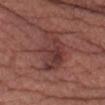Context:
About 5 mm across. The patient is a female approximately 60 years of age. Imaged with white-light lighting. A 15 mm crop from a total-body photograph taken for skin-cancer surveillance. An algorithmic analysis of the crop reported a footprint of about 13 mm² and a symmetry-axis asymmetry near 0.45. And it measured about 8 CIELAB-L* units darker than the surrounding skin and a normalized border contrast of about 7. The analysis additionally found internal color variation of about 4.5 on a 0–10 scale and peripheral color asymmetry of about 1.5. The analysis additionally found a classifier nevus-likeness of about 10/100. The lesion is located on the left thigh.